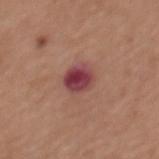notes: no biopsy performed (imaged during a skin exam); patient: female, aged approximately 50; acquisition: ~15 mm tile from a whole-body skin photo; location: the upper back.